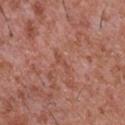The lesion was tiled from a total-body skin photograph and was not biopsied.
Cropped from a total-body skin-imaging series; the visible field is about 15 mm.
From the chest.
A male patient, aged 43–47.
The lesion-visualizer software estimated an area of roughly 2.5 mm². It also reported an automated nevus-likeness rating near 0 out of 100.